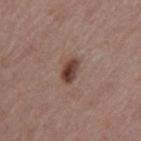| key | value |
|---|---|
| follow-up | no biopsy performed (imaged during a skin exam) |
| image source | ~15 mm tile from a whole-body skin photo |
| automated metrics | a lesion area of about 4.5 mm², an outline eccentricity of about 0.8 (0 = round, 1 = elongated), and a shape-asymmetry score of about 0.25 (0 = symmetric); a nevus-likeness score of about 95/100 and a lesion-detection confidence of about 100/100 |
| lighting | white-light |
| anatomic site | the left upper arm |
| subject | male, aged around 55 |